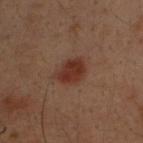A male subject, aged 28–32. From the upper back. The recorded lesion diameter is about 3.5 mm. A 15 mm crop from a total-body photograph taken for skin-cancer surveillance.